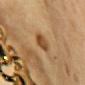Impression:
Imaged during a routine full-body skin examination; the lesion was not biopsied and no histopathology is available.
Image and clinical context:
This is a cross-polarized tile. Located on the chest. A female subject, approximately 30 years of age. A 15 mm close-up tile from a total-body photography series done for melanoma screening.